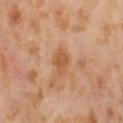Recorded during total-body skin imaging; not selected for excision or biopsy.
Captured under cross-polarized illumination.
A 15 mm close-up tile from a total-body photography series done for melanoma screening.
Longest diameter approximately 3 mm.
The subject is a female aged 53–57.
From the abdomen.